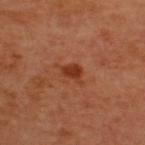• biopsy status — total-body-photography surveillance lesion; no biopsy
• location — the upper back
• diameter — ~2.5 mm (longest diameter)
• image — total-body-photography crop, ~15 mm field of view
• tile lighting — cross-polarized illumination
• image-analysis metrics — a lesion area of about 3.5 mm², a shape eccentricity near 0.75, and a shape-asymmetry score of about 0.35 (0 = symmetric); an average lesion color of about L≈37 a*≈29 b*≈35 (CIELAB), about 10 CIELAB-L* units darker than the surrounding skin, and a normalized border contrast of about 8.5; a border-irregularity rating of about 3/10, internal color variation of about 1 on a 0–10 scale, and peripheral color asymmetry of about 0.5; a detector confidence of about 100 out of 100 that the crop contains a lesion
• subject — male, aged 48–52